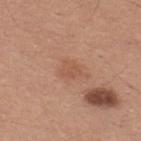| feature | finding |
|---|---|
| follow-up | no biopsy performed (imaged during a skin exam) |
| body site | the back |
| patient | male, approximately 30 years of age |
| diameter | about 2.5 mm |
| tile lighting | white-light |
| imaging modality | total-body-photography crop, ~15 mm field of view |